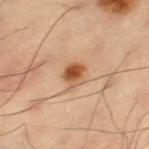This lesion was catalogued during total-body skin photography and was not selected for biopsy. The patient is a male in their 60s. From the left thigh. A lesion tile, about 15 mm wide, cut from a 3D total-body photograph.